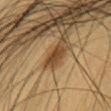Captured during whole-body skin photography for melanoma surveillance; the lesion was not biopsied.